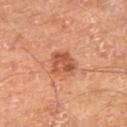biopsy_status: not biopsied; imaged during a skin examination
site: right thigh
image:
  source: total-body photography crop
  field_of_view_mm: 15
automated_metrics:
  area_mm2_approx: 7.0
  eccentricity: 0.45
  shape_asymmetry: 0.25
  border_irregularity_0_10: 2.5
  color_variation_0_10: 4.5
  peripheral_color_asymmetry: 1.5
lighting: cross-polarized
lesion_size:
  long_diameter_mm_approx: 3.0
patient:
  sex: male
  age_approx: 65